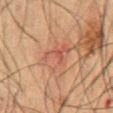{"biopsy_status": "not biopsied; imaged during a skin examination", "image": {"source": "total-body photography crop", "field_of_view_mm": 15}, "lighting": "cross-polarized", "site": "abdomen", "lesion_size": {"long_diameter_mm_approx": 4.5}, "patient": {"sex": "male", "age_approx": 50}}